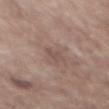The lesion was photographed on a routine skin check and not biopsied; there is no pathology result. On the mid back. A male subject, aged 68 to 72. A region of skin cropped from a whole-body photographic capture, roughly 15 mm wide.